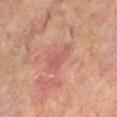Clinical impression:
This lesion was catalogued during total-body skin photography and was not selected for biopsy.
Background:
This is a cross-polarized tile. Located on the left lower leg. A male patient, aged around 65. Approximately 4 mm at its widest. Cropped from a total-body skin-imaging series; the visible field is about 15 mm.